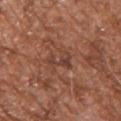Findings:
– biopsy status: no biopsy performed (imaged during a skin exam)
– patient: male, in their mid- to late 40s
– image: ~15 mm crop, total-body skin-cancer survey
– body site: the left upper arm